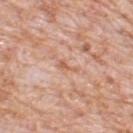About 2.5 mm across. A male subject aged 78–82. A close-up tile cropped from a whole-body skin photograph, about 15 mm across. Automated image analysis of the tile measured a lesion area of about 2.5 mm², a shape eccentricity near 0.9, and a shape-asymmetry score of about 0.6 (0 = symmetric). And it measured a mean CIELAB color near L≈61 a*≈23 b*≈33, about 7 CIELAB-L* units darker than the surrounding skin, and a normalized lesion–skin contrast near 5.5. And it measured a border-irregularity rating of about 6/10 and a within-lesion color-variation index near 0/10. It also reported an automated nevus-likeness rating near 0 out of 100 and a lesion-detection confidence of about 95/100. Located on the upper back. The tile uses white-light illumination.